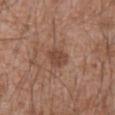Q: Was a biopsy performed?
A: total-body-photography surveillance lesion; no biopsy
Q: What kind of image is this?
A: ~15 mm tile from a whole-body skin photo
Q: What is the anatomic site?
A: the mid back
Q: How large is the lesion?
A: ≈3 mm
Q: Patient demographics?
A: male, aged 58–62
Q: Automated lesion metrics?
A: an outline eccentricity of about 0.65 (0 = round, 1 = elongated) and two-axis asymmetry of about 0.2; a mean CIELAB color near L≈45 a*≈21 b*≈28 and roughly 9 lightness units darker than nearby skin
Q: What lighting was used for the tile?
A: white-light